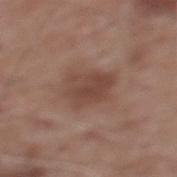{"biopsy_status": "not biopsied; imaged during a skin examination", "site": "mid back", "image": {"source": "total-body photography crop", "field_of_view_mm": 15}, "lighting": "white-light", "lesion_size": {"long_diameter_mm_approx": 4.5}, "patient": {"sex": "male", "age_approx": 60}, "automated_metrics": {"eccentricity": 0.75, "shape_asymmetry": 0.3, "border_irregularity_0_10": 3.0, "peripheral_color_asymmetry": 1.0, "lesion_detection_confidence_0_100": 100}}